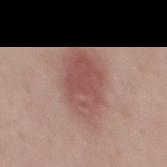<tbp_lesion>
<biopsy_status>not biopsied; imaged during a skin examination</biopsy_status>
<automated_metrics>
  <eccentricity>0.75</eccentricity>
  <shape_asymmetry>0.2</shape_asymmetry>
</automated_metrics>
<lighting>white-light</lighting>
<lesion_size>
  <long_diameter_mm_approx>7.0</long_diameter_mm_approx>
</lesion_size>
<site>mid back</site>
<image>
  <source>total-body photography crop</source>
  <field_of_view_mm>15</field_of_view_mm>
</image>
<patient>
  <sex>male</sex>
  <age_approx>45</age_approx>
</patient>
</tbp_lesion>